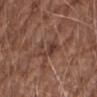The lesion was photographed on a routine skin check and not biopsied; there is no pathology result. A male patient about 70 years old. This is a white-light tile. From the right forearm. A roughly 15 mm field-of-view crop from a total-body skin photograph. An algorithmic analysis of the crop reported a footprint of about 6.5 mm², an eccentricity of roughly 0.85, and a symmetry-axis asymmetry near 0.35. The software also gave roughly 8 lightness units darker than nearby skin. The analysis additionally found a within-lesion color-variation index near 3/10 and a peripheral color-asymmetry measure near 1. Measured at roughly 4 mm in maximum diameter.